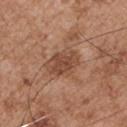* follow-up · imaged on a skin check; not biopsied
* image · ~15 mm tile from a whole-body skin photo
* site · the front of the torso
* subject · male, in their mid-50s
* illumination · white-light illumination
* size · ≈4.5 mm